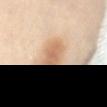Q: Is there a histopathology result?
A: total-body-photography surveillance lesion; no biopsy
Q: What lighting was used for the tile?
A: cross-polarized illumination
Q: What is the anatomic site?
A: the lower back
Q: How was this image acquired?
A: ~15 mm tile from a whole-body skin photo
Q: Automated lesion metrics?
A: a lesion color around L≈68 a*≈19 b*≈35 in CIELAB and a normalized border contrast of about 7; a peripheral color-asymmetry measure near 1; an automated nevus-likeness rating near 100 out of 100 and a lesion-detection confidence of about 100/100
Q: Who is the patient?
A: female, in their 40s